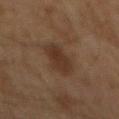Impression: No biopsy was performed on this lesion — it was imaged during a full skin examination and was not determined to be concerning. Acquisition and patient details: The lesion's longest dimension is about 5 mm. Imaged with cross-polarized lighting. The lesion-visualizer software estimated roughly 7 lightness units darker than nearby skin and a normalized lesion–skin contrast near 7.5. The software also gave border irregularity of about 2.5 on a 0–10 scale and radial color variation of about 1. A male subject, aged around 60. The lesion is on the mid back. Cropped from a total-body skin-imaging series; the visible field is about 15 mm.